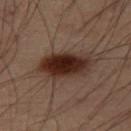Notes:
* follow-up · no biopsy performed (imaged during a skin exam)
* location · the right thigh
* lesion size · about 6 mm
* acquisition · 15 mm crop, total-body photography
* patient · male, aged 53–57
* illumination · cross-polarized